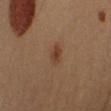Impression:
The lesion was photographed on a routine skin check and not biopsied; there is no pathology result.
Clinical summary:
A male subject aged approximately 40. The lesion's longest dimension is about 2.5 mm. Imaged with cross-polarized lighting. Located on the right upper arm. Cropped from a whole-body photographic skin survey; the tile spans about 15 mm. Automated tile analysis of the lesion measured border irregularity of about 2 on a 0–10 scale, a within-lesion color-variation index near 3/10, and radial color variation of about 1.